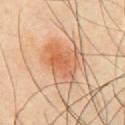Acquisition and patient details: Cropped from a total-body skin-imaging series; the visible field is about 15 mm. Approximately 5 mm at its widest. Automated image analysis of the tile measured a lesion area of about 17 mm² and an eccentricity of roughly 0.65. The software also gave a nevus-likeness score of about 100/100 and a lesion-detection confidence of about 100/100. A male patient in their 50s. From the chest.